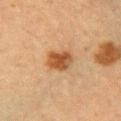biopsy_status: not biopsied; imaged during a skin examination
patient:
  sex: female
  age_approx: 55
image:
  source: total-body photography crop
  field_of_view_mm: 15
lesion_size:
  long_diameter_mm_approx: 3.5
site: right upper arm
lighting: cross-polarized
automated_metrics:
  eccentricity: 0.7
  vs_skin_contrast_norm: 9.0
  nevus_likeness_0_100: 95